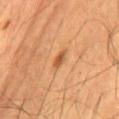Imaged during a routine full-body skin examination; the lesion was not biopsied and no histopathology is available. A lesion tile, about 15 mm wide, cut from a 3D total-body photograph. Approximately 3 mm at its widest. The lesion is located on the abdomen. A male subject roughly 60 years of age. The tile uses cross-polarized illumination. The total-body-photography lesion software estimated an average lesion color of about L≈51 a*≈24 b*≈38 (CIELAB), about 10 CIELAB-L* units darker than the surrounding skin, and a normalized lesion–skin contrast near 7. It also reported internal color variation of about 1 on a 0–10 scale and radial color variation of about 0. The analysis additionally found an automated nevus-likeness rating near 85 out of 100 and lesion-presence confidence of about 100/100.